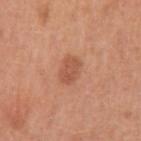Assessment: The lesion was tiled from a total-body skin photograph and was not biopsied. Clinical summary: Automated image analysis of the tile measured a footprint of about 5 mm² and an outline eccentricity of about 0.65 (0 = round, 1 = elongated). The analysis additionally found a border-irregularity rating of about 2/10 and radial color variation of about 1. The tile uses white-light illumination. The recorded lesion diameter is about 2.5 mm. The lesion is on the left upper arm. A close-up tile cropped from a whole-body skin photograph, about 15 mm across. A male patient in their mid- to late 50s.